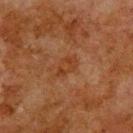Assessment: Recorded during total-body skin imaging; not selected for excision or biopsy. Background: The lesion's longest dimension is about 3 mm. A male subject about 80 years old. Located on the upper back. This image is a 15 mm lesion crop taken from a total-body photograph. An algorithmic analysis of the crop reported a lesion area of about 4 mm², an eccentricity of roughly 0.8, and two-axis asymmetry of about 0.25. And it measured a mean CIELAB color near L≈31 a*≈20 b*≈30, a lesion–skin lightness drop of about 5, and a normalized lesion–skin contrast near 6.5.